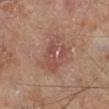follow-up = total-body-photography surveillance lesion; no biopsy
illumination = cross-polarized
subject = male, aged 63–67
location = the right lower leg
image = total-body-photography crop, ~15 mm field of view